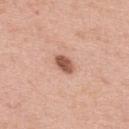Captured during whole-body skin photography for melanoma surveillance; the lesion was not biopsied.
A male patient, roughly 40 years of age.
Imaged with white-light lighting.
Located on the upper back.
Longest diameter approximately 2.5 mm.
Cropped from a total-body skin-imaging series; the visible field is about 15 mm.
The lesion-visualizer software estimated an average lesion color of about L≈55 a*≈24 b*≈30 (CIELAB). And it measured a nevus-likeness score of about 85/100 and a detector confidence of about 100 out of 100 that the crop contains a lesion.